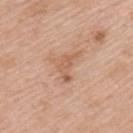notes = no biopsy performed (imaged during a skin exam) | anatomic site = the left upper arm | patient = male, aged 58 to 62 | tile lighting = white-light | imaging modality = ~15 mm crop, total-body skin-cancer survey | lesion size = ~4 mm (longest diameter) | TBP lesion metrics = a border-irregularity index near 7.5/10 and internal color variation of about 1.5 on a 0–10 scale; a nevus-likeness score of about 0/100 and a lesion-detection confidence of about 100/100.